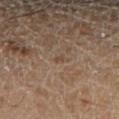| feature | finding |
|---|---|
| biopsy status | no biopsy performed (imaged during a skin exam) |
| patient | male, in their mid- to late 50s |
| tile lighting | cross-polarized illumination |
| anatomic site | the right lower leg |
| lesion size | about 1 mm |
| image source | ~15 mm tile from a whole-body skin photo |